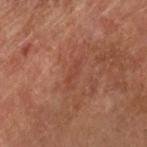Captured during whole-body skin photography for melanoma surveillance; the lesion was not biopsied.